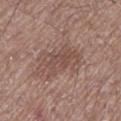Assessment: Part of a total-body skin-imaging series; this lesion was reviewed on a skin check and was not flagged for biopsy. Acquisition and patient details: Captured under white-light illumination. Cropped from a total-body skin-imaging series; the visible field is about 15 mm. The lesion is on the left lower leg. A male patient, roughly 70 years of age. About 5 mm across.